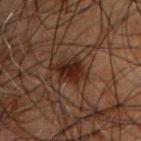Assessment: Part of a total-body skin-imaging series; this lesion was reviewed on a skin check and was not flagged for biopsy. Background: A close-up tile cropped from a whole-body skin photograph, about 15 mm across. This is a cross-polarized tile. The total-body-photography lesion software estimated an average lesion color of about L≈18 a*≈15 b*≈20 (CIELAB) and a normalized lesion–skin contrast near 10.5. The patient is a male approximately 50 years of age. The lesion is on the chest. Approximately 4 mm at its widest.